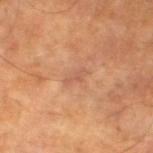{"biopsy_status": "not biopsied; imaged during a skin examination", "lighting": "cross-polarized", "image": {"source": "total-body photography crop", "field_of_view_mm": 15}, "lesion_size": {"long_diameter_mm_approx": 3.0}, "site": "left lower leg", "automated_metrics": {"border_irregularity_0_10": 3.5, "color_variation_0_10": 0.0, "peripheral_color_asymmetry": 0.0}, "patient": {"sex": "male", "age_approx": 65}}